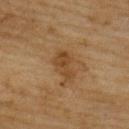| field | value |
|---|---|
| notes | catalogued during a skin exam; not biopsied |